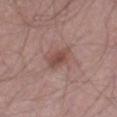Impression: Imaged during a routine full-body skin examination; the lesion was not biopsied and no histopathology is available. Clinical summary: Captured under white-light illumination. On the right thigh. A male subject roughly 70 years of age. A region of skin cropped from a whole-body photographic capture, roughly 15 mm wide.